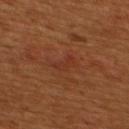Assessment:
Captured during whole-body skin photography for melanoma surveillance; the lesion was not biopsied.
Acquisition and patient details:
A male patient, aged 43–47. Cropped from a total-body skin-imaging series; the visible field is about 15 mm. The total-body-photography lesion software estimated border irregularity of about 5 on a 0–10 scale, a color-variation rating of about 0/10, and radial color variation of about 0. The software also gave a lesion-detection confidence of about 100/100. On the upper back. This is a cross-polarized tile.